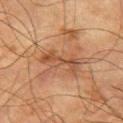notes: catalogued during a skin exam; not biopsied | tile lighting: cross-polarized illumination | patient: male, in their mid-70s | automated metrics: a symmetry-axis asymmetry near 0.5; a mean CIELAB color near L≈42 a*≈21 b*≈30, about 8 CIELAB-L* units darker than the surrounding skin, and a lesion-to-skin contrast of about 6.5 (normalized; higher = more distinct) | anatomic site: the right upper arm | size: about 4.5 mm | image source: total-body-photography crop, ~15 mm field of view.